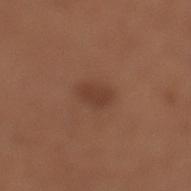Imaged during a routine full-body skin examination; the lesion was not biopsied and no histopathology is available. Longest diameter approximately 3 mm. Captured under white-light illumination. On the left lower leg. The subject is a female roughly 55 years of age. A 15 mm close-up tile from a total-body photography series done for melanoma screening.